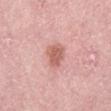Case summary:
* image source: total-body-photography crop, ~15 mm field of view
* anatomic site: the right lower leg
* subject: female, aged around 65
* automated lesion analysis: an area of roughly 6 mm², a shape eccentricity near 0.6, and two-axis asymmetry of about 0.3; a mean CIELAB color near L≈60 a*≈26 b*≈26, a lesion–skin lightness drop of about 11, and a normalized border contrast of about 7; a nevus-likeness score of about 45/100 and a detector confidence of about 100 out of 100 that the crop contains a lesion